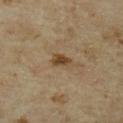{
  "biopsy_status": "not biopsied; imaged during a skin examination",
  "lighting": "cross-polarized",
  "site": "left upper arm",
  "patient": {
    "sex": "female",
    "age_approx": 35
  },
  "image": {
    "source": "total-body photography crop",
    "field_of_view_mm": 15
  },
  "lesion_size": {
    "long_diameter_mm_approx": 3.0
  }
}